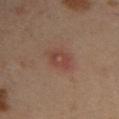Recorded during total-body skin imaging; not selected for excision or biopsy.
Cropped from a total-body skin-imaging series; the visible field is about 15 mm.
The patient is a male about 40 years old.
The lesion is located on the right upper arm.
The tile uses cross-polarized illumination.